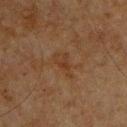body site — the front of the torso | acquisition — ~15 mm crop, total-body skin-cancer survey | patient — male, in their mid-60s | size — about 3 mm | lighting — cross-polarized.